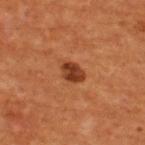follow-up — total-body-photography surveillance lesion; no biopsy | patient — male, approximately 55 years of age | acquisition — ~15 mm tile from a whole-body skin photo | lesion diameter — about 3 mm | anatomic site — the upper back | image-analysis metrics — a mean CIELAB color near L≈38 a*≈27 b*≈35, a lesion–skin lightness drop of about 13, and a lesion-to-skin contrast of about 10 (normalized; higher = more distinct).